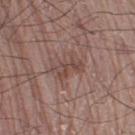No biopsy was performed on this lesion — it was imaged during a full skin examination and was not determined to be concerning. The lesion's longest dimension is about 4 mm. Imaged with white-light lighting. A close-up tile cropped from a whole-body skin photograph, about 15 mm across. On the right thigh. The subject is a male in their mid- to late 70s.